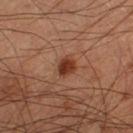{
  "site": "left thigh",
  "image": {
    "source": "total-body photography crop",
    "field_of_view_mm": 15
  },
  "lesion_size": {
    "long_diameter_mm_approx": 2.5
  },
  "patient": {
    "sex": "male",
    "age_approx": 60
  },
  "automated_metrics": {
    "cielab_L": 37,
    "cielab_a": 25,
    "cielab_b": 30,
    "vs_skin_darker_L": 12.0,
    "vs_skin_contrast_norm": 10.0,
    "nevus_likeness_0_100": 95,
    "lesion_detection_confidence_0_100": 100
  }
}